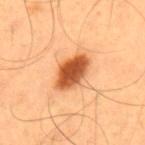biopsy status = no biopsy performed (imaged during a skin exam) | body site = the upper back | image = ~15 mm tile from a whole-body skin photo | subject = male, about 55 years old | automated metrics = an average lesion color of about L≈55 a*≈30 b*≈43 (CIELAB), a lesion–skin lightness drop of about 19, and a normalized border contrast of about 12; border irregularity of about 1.5 on a 0–10 scale, internal color variation of about 5 on a 0–10 scale, and a peripheral color-asymmetry measure near 1.5; a nevus-likeness score of about 100/100 and a detector confidence of about 100 out of 100 that the crop contains a lesion | tile lighting = cross-polarized illumination.